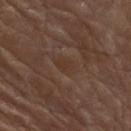<record>
<biopsy_status>not biopsied; imaged during a skin examination</biopsy_status>
<automated_metrics>
  <eccentricity>0.8</eccentricity>
  <shape_asymmetry>0.2</shape_asymmetry>
  <cielab_L>34</cielab_L>
  <cielab_a>16</cielab_a>
  <cielab_b>25</cielab_b>
  <vs_skin_contrast_norm>5.0</vs_skin_contrast_norm>
</automated_metrics>
<site>front of the torso</site>
<lesion_size>
  <long_diameter_mm_approx>3.0</long_diameter_mm_approx>
</lesion_size>
<lighting>white-light</lighting>
<patient>
  <sex>male</sex>
  <age_approx>80</age_approx>
</patient>
<image>
  <source>total-body photography crop</source>
  <field_of_view_mm>15</field_of_view_mm>
</image>
</record>